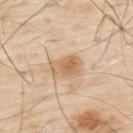| key | value |
|---|---|
| biopsy status | catalogued during a skin exam; not biopsied |
| automated lesion analysis | a border-irregularity rating of about 2.5/10 and peripheral color asymmetry of about 1.5; an automated nevus-likeness rating near 50 out of 100 and lesion-presence confidence of about 100/100 |
| lesion size | ≈3.5 mm |
| subject | male, aged approximately 80 |
| acquisition | ~15 mm crop, total-body skin-cancer survey |
| site | the upper back |